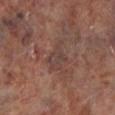Captured during whole-body skin photography for melanoma surveillance; the lesion was not biopsied.
The tile uses cross-polarized illumination.
About 4 mm across.
The patient is a male aged 63–67.
The lesion is on the left lower leg.
A 15 mm close-up extracted from a 3D total-body photography capture.